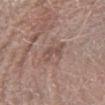{"site": "arm", "lighting": "white-light", "patient": {"sex": "male", "age_approx": 80}, "image": {"source": "total-body photography crop", "field_of_view_mm": 15}}